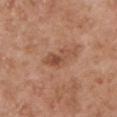  biopsy_status: not biopsied; imaged during a skin examination
  automated_metrics:
    area_mm2_approx: 6.0
    eccentricity: 0.85
    shape_asymmetry: 0.4
    vs_skin_darker_L: 9.0
  site: left upper arm
  image:
    source: total-body photography crop
    field_of_view_mm: 15
  lighting: white-light
  patient:
    sex: male
    age_approx: 55